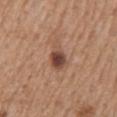About 3.5 mm across.
A male patient about 70 years old.
The tile uses white-light illumination.
The lesion is on the mid back.
An algorithmic analysis of the crop reported a footprint of about 6 mm² and two-axis asymmetry of about 0.3. It also reported a border-irregularity rating of about 3/10, a within-lesion color-variation index near 6.5/10, and peripheral color asymmetry of about 2. It also reported a detector confidence of about 100 out of 100 that the crop contains a lesion.
A 15 mm close-up extracted from a 3D total-body photography capture.